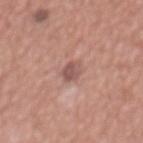Imaged during a routine full-body skin examination; the lesion was not biopsied and no histopathology is available. A male patient, aged around 45. The recorded lesion diameter is about 2.5 mm. The lesion-visualizer software estimated border irregularity of about 2 on a 0–10 scale, internal color variation of about 2 on a 0–10 scale, and radial color variation of about 0.5. A lesion tile, about 15 mm wide, cut from a 3D total-body photograph. The lesion is on the front of the torso. Imaged with white-light lighting.